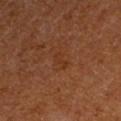Notes:
* workup: catalogued during a skin exam; not biopsied
* imaging modality: ~15 mm crop, total-body skin-cancer survey
* body site: the left upper arm
* size: ~2.5 mm (longest diameter)
* subject: female
* illumination: cross-polarized